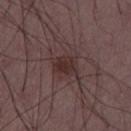notes — no biopsy performed (imaged during a skin exam) | image — ~15 mm crop, total-body skin-cancer survey | lesion size — ~3 mm (longest diameter) | lighting — white-light | patient — male, aged approximately 50 | body site — the left thigh.